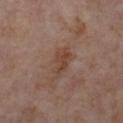Case summary:
* follow-up: imaged on a skin check; not biopsied
* automated metrics: an average lesion color of about L≈43 a*≈19 b*≈27 (CIELAB); border irregularity of about 3.5 on a 0–10 scale, a color-variation rating of about 2.5/10, and radial color variation of about 1
* illumination: cross-polarized
* location: the left thigh
* subject: female, aged around 55
* image source: ~15 mm crop, total-body skin-cancer survey
* lesion size: about 3.5 mm